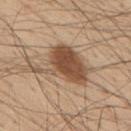biopsy_status: not biopsied; imaged during a skin examination
image:
  source: total-body photography crop
  field_of_view_mm: 15
automated_metrics:
  cielab_L: 50
  cielab_a: 18
  cielab_b: 31
  vs_skin_darker_L: 14.0
  vs_skin_contrast_norm: 10.0
site: upper back
patient:
  sex: male
  age_approx: 55
lesion_size:
  long_diameter_mm_approx: 6.5